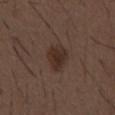Assessment: Captured during whole-body skin photography for melanoma surveillance; the lesion was not biopsied. Context: The subject is a male approximately 50 years of age. A lesion tile, about 15 mm wide, cut from a 3D total-body photograph. This is a white-light tile. From the abdomen. About 3.5 mm across.